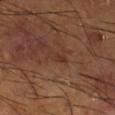Q: Lesion size?
A: about 2.5 mm
Q: What is the anatomic site?
A: the right lower leg
Q: What kind of image is this?
A: total-body-photography crop, ~15 mm field of view
Q: Patient demographics?
A: male, in their mid-60s